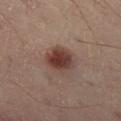Notes:
– biopsy status: no biopsy performed (imaged during a skin exam)
– lesion diameter: about 3.5 mm
– anatomic site: the left thigh
– automated metrics: an average lesion color of about L≈34 a*≈17 b*≈20 (CIELAB), a lesion–skin lightness drop of about 11, and a normalized lesion–skin contrast near 10; a color-variation rating of about 4/10 and a peripheral color-asymmetry measure near 1
– illumination: cross-polarized
– subject: male, aged approximately 50
– image: total-body-photography crop, ~15 mm field of view